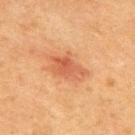Impression: Captured during whole-body skin photography for melanoma surveillance; the lesion was not biopsied. Clinical summary: The lesion is located on the back. This is a cross-polarized tile. Longest diameter approximately 5 mm. A male patient, approximately 50 years of age. This image is a 15 mm lesion crop taken from a total-body photograph.